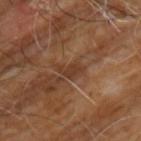| field | value |
|---|---|
| workup | catalogued during a skin exam; not biopsied |
| anatomic site | the chest |
| patient | male, about 60 years old |
| image-analysis metrics | an area of roughly 4 mm², an outline eccentricity of about 0.8 (0 = round, 1 = elongated), and a shape-asymmetry score of about 0.35 (0 = symmetric) |
| illumination | cross-polarized illumination |
| acquisition | 15 mm crop, total-body photography |
| lesion size | about 3 mm |